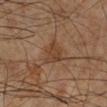Q: Is there a histopathology result?
A: catalogued during a skin exam; not biopsied
Q: How large is the lesion?
A: ≈3 mm
Q: How was this image acquired?
A: ~15 mm tile from a whole-body skin photo
Q: What are the patient's age and sex?
A: male, aged 58 to 62
Q: How was the tile lit?
A: cross-polarized illumination
Q: What did automated image analysis measure?
A: a lesion color around L≈36 a*≈17 b*≈27 in CIELAB, about 6 CIELAB-L* units darker than the surrounding skin, and a lesion-to-skin contrast of about 6 (normalized; higher = more distinct); a border-irregularity rating of about 3.5/10 and a within-lesion color-variation index near 2.5/10; a nevus-likeness score of about 0/100
Q: Where on the body is the lesion?
A: the left lower leg